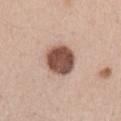This lesion was catalogued during total-body skin photography and was not selected for biopsy.
Cropped from a total-body skin-imaging series; the visible field is about 15 mm.
Automated tile analysis of the lesion measured a lesion area of about 12 mm², a shape eccentricity near 0.7, and a shape-asymmetry score of about 0.15 (0 = symmetric).
The lesion is on the left upper arm.
The tile uses white-light illumination.
A female subject aged 38 to 42.
The lesion's longest dimension is about 4.5 mm.